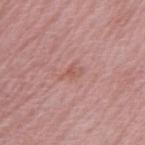Captured during whole-body skin photography for melanoma surveillance; the lesion was not biopsied.
On the arm.
A region of skin cropped from a whole-body photographic capture, roughly 15 mm wide.
A female subject aged around 60.